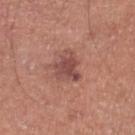Part of a total-body skin-imaging series; this lesion was reviewed on a skin check and was not flagged for biopsy.
Longest diameter approximately 4 mm.
The tile uses white-light illumination.
The lesion is on the right lower leg.
An algorithmic analysis of the crop reported a nevus-likeness score of about 45/100 and a lesion-detection confidence of about 100/100.
A male subject, aged 68–72.
A 15 mm close-up tile from a total-body photography series done for melanoma screening.